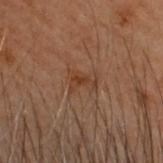Q: Was a biopsy performed?
A: no biopsy performed (imaged during a skin exam)
Q: How was the tile lit?
A: cross-polarized illumination
Q: How was this image acquired?
A: 15 mm crop, total-body photography
Q: Patient demographics?
A: male, aged around 55
Q: Lesion location?
A: the head or neck
Q: Automated lesion metrics?
A: a lesion area of about 4 mm², a shape eccentricity near 0.75, and two-axis asymmetry of about 0.45; an average lesion color of about L≈31 a*≈17 b*≈25 (CIELAB), about 5 CIELAB-L* units darker than the surrounding skin, and a normalized border contrast of about 6; a border-irregularity index near 5/10, a within-lesion color-variation index near 1/10, and a peripheral color-asymmetry measure near 0.5; a nevus-likeness score of about 0/100 and lesion-presence confidence of about 100/100